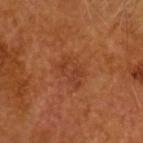Q: Was this lesion biopsied?
A: catalogued during a skin exam; not biopsied
Q: Where on the body is the lesion?
A: the head or neck
Q: What kind of image is this?
A: ~15 mm crop, total-body skin-cancer survey
Q: What is the lesion's diameter?
A: ≈4 mm
Q: Who is the patient?
A: male, roughly 65 years of age